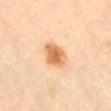This lesion was catalogued during total-body skin photography and was not selected for biopsy. The patient is a female in their 60s. Measured at roughly 3.5 mm in maximum diameter. This is a cross-polarized tile. On the abdomen. A roughly 15 mm field-of-view crop from a total-body skin photograph. The total-body-photography lesion software estimated a mean CIELAB color near L≈70 a*≈24 b*≈43 and a lesion-to-skin contrast of about 9 (normalized; higher = more distinct). And it measured a border-irregularity index near 1.5/10 and radial color variation of about 1. The software also gave an automated nevus-likeness rating near 100 out of 100 and a detector confidence of about 100 out of 100 that the crop contains a lesion.